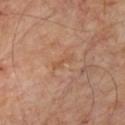No biopsy was performed on this lesion — it was imaged during a full skin examination and was not determined to be concerning. A 15 mm crop from a total-body photograph taken for skin-cancer surveillance. A male subject about 55 years old. An algorithmic analysis of the crop reported a lesion color around L≈52 a*≈21 b*≈33 in CIELAB, about 5 CIELAB-L* units darker than the surrounding skin, and a lesion-to-skin contrast of about 5 (normalized; higher = more distinct). The lesion is located on the chest. The lesion's longest dimension is about 3 mm.